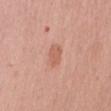A 15 mm close-up tile from a total-body photography series done for melanoma screening.
A female patient, aged 38–42.
Automated image analysis of the tile measured a lesion area of about 4.5 mm² and two-axis asymmetry of about 0.2. It also reported an average lesion color of about L≈60 a*≈25 b*≈31 (CIELAB), a lesion–skin lightness drop of about 8, and a normalized lesion–skin contrast near 6. The software also gave a border-irregularity index near 2/10, a color-variation rating of about 1.5/10, and peripheral color asymmetry of about 0.5. It also reported a nevus-likeness score of about 45/100 and a lesion-detection confidence of about 100/100.
The recorded lesion diameter is about 2.5 mm.
On the right upper arm.
The tile uses white-light illumination.